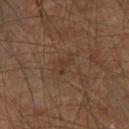* notes · no biopsy performed (imaged during a skin exam)